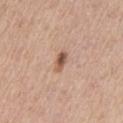Recorded during total-body skin imaging; not selected for excision or biopsy. A female subject aged 38–42. The lesion is on the left upper arm. An algorithmic analysis of the crop reported a mean CIELAB color near L≈55 a*≈20 b*≈29 and a lesion-to-skin contrast of about 8.5 (normalized; higher = more distinct). And it measured a border-irregularity index near 2/10 and a within-lesion color-variation index near 6/10. And it measured lesion-presence confidence of about 100/100. A lesion tile, about 15 mm wide, cut from a 3D total-body photograph. Measured at roughly 2.5 mm in maximum diameter.